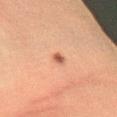| feature | finding |
|---|---|
| biopsy status | imaged on a skin check; not biopsied |
| imaging modality | ~15 mm tile from a whole-body skin photo |
| site | the right forearm |
| lesion size | about 1.5 mm |
| subject | male, about 40 years old |
| lighting | cross-polarized illumination |